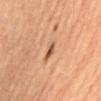| feature | finding |
|---|---|
| patient | female, aged 58 to 62 |
| anatomic site | the mid back |
| lesion size | about 2.5 mm |
| automated lesion analysis | a footprint of about 3 mm² and an eccentricity of roughly 0.85; a nevus-likeness score of about 20/100 and lesion-presence confidence of about 100/100 |
| image source | 15 mm crop, total-body photography |